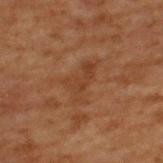Assessment: The lesion was tiled from a total-body skin photograph and was not biopsied.